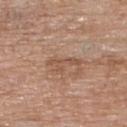Part of a total-body skin-imaging series; this lesion was reviewed on a skin check and was not flagged for biopsy.
A region of skin cropped from a whole-body photographic capture, roughly 15 mm wide.
Located on the upper back.
The subject is a female aged approximately 75.
Captured under white-light illumination.